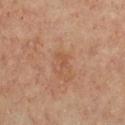* notes — catalogued during a skin exam; not biopsied
* size — ≈2.5 mm
* image source — total-body-photography crop, ~15 mm field of view
* subject — female, aged 43–47
* location — the chest
* tile lighting — cross-polarized illumination
* automated lesion analysis — a border-irregularity index near 7/10, a within-lesion color-variation index near 0/10, and a peripheral color-asymmetry measure near 0; an automated nevus-likeness rating near 0 out of 100